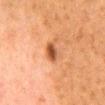Q: Was a biopsy performed?
A: catalogued during a skin exam; not biopsied
Q: What are the patient's age and sex?
A: female, roughly 50 years of age
Q: How was this image acquired?
A: ~15 mm crop, total-body skin-cancer survey
Q: Lesion location?
A: the back
Q: Illumination type?
A: cross-polarized illumination